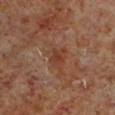Findings:
- biopsy status — imaged on a skin check; not biopsied
- location — the left lower leg
- patient — male, about 60 years old
- image — 15 mm crop, total-body photography
- lighting — cross-polarized illumination
- lesion diameter — ~3 mm (longest diameter)